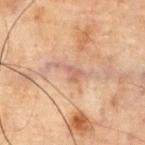The lesion was photographed on a routine skin check and not biopsied; there is no pathology result.
A male subject, aged 68–72.
The lesion is located on the chest.
A roughly 15 mm field-of-view crop from a total-body skin photograph.